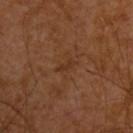- biopsy status · total-body-photography surveillance lesion; no biopsy
- image source · 15 mm crop, total-body photography
- patient · male, in their 30s
- lighting · cross-polarized illumination
- image-analysis metrics · roughly 5 lightness units darker than nearby skin and a normalized border contrast of about 5.5; a classifier nevus-likeness of about 0/100
- lesion size · about 2.5 mm
- location · the upper back